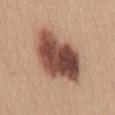Assessment:
The lesion was photographed on a routine skin check and not biopsied; there is no pathology result.
Clinical summary:
A female patient in their mid-30s. A region of skin cropped from a whole-body photographic capture, roughly 15 mm wide. Located on the mid back.